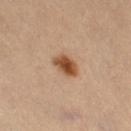workup — total-body-photography surveillance lesion; no biopsy
image-analysis metrics — a footprint of about 5.5 mm², an outline eccentricity of about 0.75 (0 = round, 1 = elongated), and two-axis asymmetry of about 0.25; a mean CIELAB color near L≈47 a*≈21 b*≈34 and about 14 CIELAB-L* units darker than the surrounding skin; border irregularity of about 2.5 on a 0–10 scale and internal color variation of about 4.5 on a 0–10 scale; an automated nevus-likeness rating near 100 out of 100 and a detector confidence of about 100 out of 100 that the crop contains a lesion
image — ~15 mm tile from a whole-body skin photo
subject — female, in their mid-30s
anatomic site — the right thigh
lesion size — ~3 mm (longest diameter)